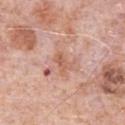Recorded during total-body skin imaging; not selected for excision or biopsy.